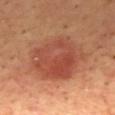Case summary:
– subject · male, in their mid-60s
– image-analysis metrics · a lesion area of about 33 mm², a shape eccentricity near 0.7, and two-axis asymmetry of about 0.15; an average lesion color of about L≈48 a*≈27 b*≈32 (CIELAB), roughly 10 lightness units darker than nearby skin, and a normalized border contrast of about 7.5; border irregularity of about 2 on a 0–10 scale, a color-variation rating of about 6/10, and radial color variation of about 2; a classifier nevus-likeness of about 95/100 and lesion-presence confidence of about 100/100
– lesion size · ~8.5 mm (longest diameter)
– acquisition · ~15 mm crop, total-body skin-cancer survey
– body site · the mid back
– lighting · cross-polarized